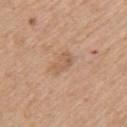Assessment: The lesion was photographed on a routine skin check and not biopsied; there is no pathology result. Clinical summary: An algorithmic analysis of the crop reported a color-variation rating of about 2.5/10 and peripheral color asymmetry of about 1.5. It also reported a classifier nevus-likeness of about 5/100. Measured at roughly 3 mm in maximum diameter. Located on the left upper arm. The tile uses white-light illumination. A lesion tile, about 15 mm wide, cut from a 3D total-body photograph. A male subject aged 58 to 62.